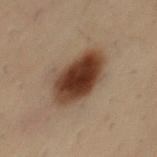  biopsy_status: not biopsied; imaged during a skin examination
  lesion_size:
    long_diameter_mm_approx: 5.5
  lighting: cross-polarized
  patient:
    sex: male
    age_approx: 30
  automated_metrics:
    area_mm2_approx: 19.0
    eccentricity: 0.7
    vs_skin_darker_L: 15.0
  image:
    source: total-body photography crop
    field_of_view_mm: 15
  site: mid back